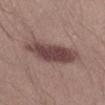Part of a total-body skin-imaging series; this lesion was reviewed on a skin check and was not flagged for biopsy.
A male subject approximately 30 years of age.
A 15 mm close-up extracted from a 3D total-body photography capture.
Automated tile analysis of the lesion measured an area of roughly 17 mm², an outline eccentricity of about 0.9 (0 = round, 1 = elongated), and a symmetry-axis asymmetry near 0.25. The analysis additionally found a mean CIELAB color near L≈43 a*≈19 b*≈18 and a normalized lesion–skin contrast near 10.5. The software also gave border irregularity of about 3 on a 0–10 scale, internal color variation of about 3 on a 0–10 scale, and a peripheral color-asymmetry measure near 1. And it measured an automated nevus-likeness rating near 85 out of 100 and a detector confidence of about 100 out of 100 that the crop contains a lesion.
The lesion's longest dimension is about 7 mm.
The tile uses white-light illumination.